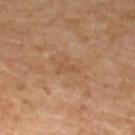The lesion was photographed on a routine skin check and not biopsied; there is no pathology result. A female patient approximately 70 years of age. On the left lower leg. A close-up tile cropped from a whole-body skin photograph, about 15 mm across.